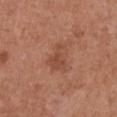Q: Is there a histopathology result?
A: catalogued during a skin exam; not biopsied
Q: Who is the patient?
A: female, in their mid-60s
Q: Where on the body is the lesion?
A: the chest
Q: How was this image acquired?
A: total-body-photography crop, ~15 mm field of view
Q: How was the tile lit?
A: white-light
Q: Lesion size?
A: ~3.5 mm (longest diameter)